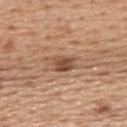From the upper back. A roughly 15 mm field-of-view crop from a total-body skin photograph. The patient is a female aged approximately 55.